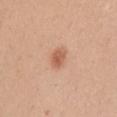<lesion>
<biopsy_status>not biopsied; imaged during a skin examination</biopsy_status>
<site>chest</site>
<lesion_size>
  <long_diameter_mm_approx>2.5</long_diameter_mm_approx>
</lesion_size>
<image>
  <source>total-body photography crop</source>
  <field_of_view_mm>15</field_of_view_mm>
</image>
<patient>
  <sex>male</sex>
  <age_approx>40</age_approx>
</patient>
<lighting>white-light</lighting>
<automated_metrics>
  <area_mm2_approx>4.5</area_mm2_approx>
  <eccentricity>0.75</eccentricity>
  <shape_asymmetry>0.25</shape_asymmetry>
  <nevus_likeness_0_100>95</nevus_likeness_0_100>
  <lesion_detection_confidence_0_100>100</lesion_detection_confidence_0_100>
</automated_metrics>
</lesion>